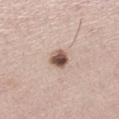Impression:
The lesion was tiled from a total-body skin photograph and was not biopsied.